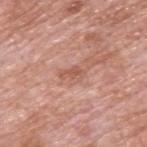<case>
<biopsy_status>not biopsied; imaged during a skin examination</biopsy_status>
<lesion_size>
  <long_diameter_mm_approx>2.5</long_diameter_mm_approx>
</lesion_size>
<lighting>white-light</lighting>
<site>upper back</site>
<patient>
  <sex>male</sex>
  <age_approx>60</age_approx>
</patient>
<image>
  <source>total-body photography crop</source>
  <field_of_view_mm>15</field_of_view_mm>
</image>
<automated_metrics>
  <area_mm2_approx>3.0</area_mm2_approx>
  <eccentricity>0.85</eccentricity>
  <shape_asymmetry>0.3</shape_asymmetry>
  <cielab_L>56</cielab_L>
  <cielab_a>24</cielab_a>
  <cielab_b>29</cielab_b>
  <vs_skin_darker_L>8.0</vs_skin_darker_L>
  <vs_skin_contrast_norm>6.0</vs_skin_contrast_norm>
</automated_metrics>
</case>